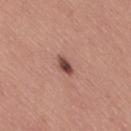This lesion was catalogued during total-body skin photography and was not selected for biopsy. Longest diameter approximately 2.5 mm. Cropped from a total-body skin-imaging series; the visible field is about 15 mm. The total-body-photography lesion software estimated an eccentricity of roughly 0.8 and two-axis asymmetry of about 0.25. And it measured about 15 CIELAB-L* units darker than the surrounding skin and a normalized border contrast of about 10.5. The analysis additionally found a border-irregularity index near 2/10 and radial color variation of about 1.5. A female patient in their 30s. From the leg.